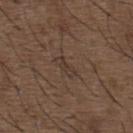Assessment: This lesion was catalogued during total-body skin photography and was not selected for biopsy. Background: The lesion's longest dimension is about 3 mm. Cropped from a whole-body photographic skin survey; the tile spans about 15 mm. A male patient aged 48 to 52. Located on the upper back.